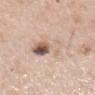The lesion was tiled from a total-body skin photograph and was not biopsied.
Cropped from a whole-body photographic skin survey; the tile spans about 15 mm.
A male patient, in their mid- to late 60s.
From the mid back.